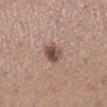automated lesion analysis: a border-irregularity index near 2/10, a color-variation rating of about 5.5/10, and radial color variation of about 2
tile lighting: white-light
site: the left lower leg
patient: female, aged around 30
imaging modality: ~15 mm tile from a whole-body skin photo
lesion size: about 2.5 mm